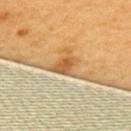<record>
  <biopsy_status>not biopsied; imaged during a skin examination</biopsy_status>
  <lesion_size>
    <long_diameter_mm_approx>3.0</long_diameter_mm_approx>
  </lesion_size>
  <image>
    <source>total-body photography crop</source>
    <field_of_view_mm>15</field_of_view_mm>
  </image>
  <lighting>cross-polarized</lighting>
  <site>upper back</site>
  <patient>
    <sex>female</sex>
    <age_approx>55</age_approx>
  </patient>
  <automated_metrics>
    <area_mm2_approx>4.5</area_mm2_approx>
    <eccentricity>0.8</eccentricity>
    <shape_asymmetry>0.3</shape_asymmetry>
    <cielab_L>59</cielab_L>
    <cielab_a>22</cielab_a>
    <cielab_b>43</cielab_b>
    <vs_skin_darker_L>12.0</vs_skin_darker_L>
    <vs_skin_contrast_norm>8.5</vs_skin_contrast_norm>
    <border_irregularity_0_10>3.0</border_irregularity_0_10>
    <color_variation_0_10>3.0</color_variation_0_10>
    <peripheral_color_asymmetry>1.0</peripheral_color_asymmetry>
    <nevus_likeness_0_100>0</nevus_likeness_0_100>
  </automated_metrics>
</record>